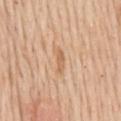Clinical impression:
Imaged during a routine full-body skin examination; the lesion was not biopsied and no histopathology is available.
Context:
Automated image analysis of the tile measured a border-irregularity index near 4.5/10, a color-variation rating of about 0/10, and a peripheral color-asymmetry measure near 0. And it measured a nevus-likeness score of about 0/100 and a detector confidence of about 100 out of 100 that the crop contains a lesion. Approximately 2.5 mm at its widest. Cropped from a whole-body photographic skin survey; the tile spans about 15 mm. A male subject approximately 60 years of age. The tile uses white-light illumination. From the mid back.